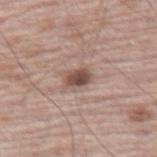Assessment: Part of a total-body skin-imaging series; this lesion was reviewed on a skin check and was not flagged for biopsy. Context: A 15 mm crop from a total-body photograph taken for skin-cancer surveillance. Approximately 2.5 mm at its widest. From the left thigh. A male subject aged 68 to 72.